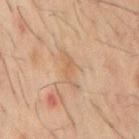Imaged during a routine full-body skin examination; the lesion was not biopsied and no histopathology is available.
The tile uses cross-polarized illumination.
From the mid back.
A close-up tile cropped from a whole-body skin photograph, about 15 mm across.
The subject is a male aged 58 to 62.
Longest diameter approximately 4 mm.
The lesion-visualizer software estimated a lesion area of about 4.5 mm², an outline eccentricity of about 0.95 (0 = round, 1 = elongated), and two-axis asymmetry of about 0.45. It also reported about 6 CIELAB-L* units darker than the surrounding skin and a lesion-to-skin contrast of about 5 (normalized; higher = more distinct). The software also gave a border-irregularity rating of about 6/10, internal color variation of about 0.5 on a 0–10 scale, and peripheral color asymmetry of about 0. And it measured a nevus-likeness score of about 0/100 and a detector confidence of about 95 out of 100 that the crop contains a lesion.